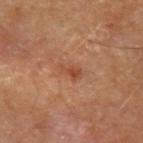{
  "biopsy_status": "not biopsied; imaged during a skin examination",
  "image": {
    "source": "total-body photography crop",
    "field_of_view_mm": 15
  },
  "lighting": "cross-polarized",
  "site": "left forearm",
  "lesion_size": {
    "long_diameter_mm_approx": 2.5
  },
  "patient": {
    "sex": "male",
    "age_approx": 70
  }
}